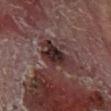The tile uses cross-polarized illumination.
A male patient in their mid- to late 50s.
A close-up tile cropped from a whole-body skin photograph, about 15 mm across.
Located on the left lower leg.